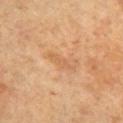notes=catalogued during a skin exam; not biopsied
lighting=cross-polarized illumination
image=~15 mm tile from a whole-body skin photo
subject=female, aged 58 to 62
location=the chest
size=~3.5 mm (longest diameter)